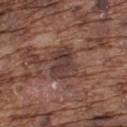workup = total-body-photography surveillance lesion; no biopsy
patient = male, aged 73–77
image = total-body-photography crop, ~15 mm field of view
site = the upper back